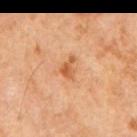Assessment: Captured during whole-body skin photography for melanoma surveillance; the lesion was not biopsied. Acquisition and patient details: Captured under cross-polarized illumination. Automated tile analysis of the lesion measured a lesion color around L≈57 a*≈26 b*≈40 in CIELAB and a normalized border contrast of about 7.5. And it measured a nevus-likeness score of about 45/100. On the right upper arm. The patient is a male in their mid- to late 60s. The lesion's longest dimension is about 2.5 mm. A roughly 15 mm field-of-view crop from a total-body skin photograph.